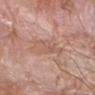Impression:
Imaged during a routine full-body skin examination; the lesion was not biopsied and no histopathology is available.
Background:
Imaged with white-light lighting. A lesion tile, about 15 mm wide, cut from a 3D total-body photograph. Located on the right forearm. Automated tile analysis of the lesion measured an area of roughly 7.5 mm², an eccentricity of roughly 0.9, and a symmetry-axis asymmetry near 0.3. It also reported a mean CIELAB color near L≈58 a*≈21 b*≈27, about 7 CIELAB-L* units darker than the surrounding skin, and a lesion-to-skin contrast of about 5 (normalized; higher = more distinct). The analysis additionally found a border-irregularity rating of about 5/10, a within-lesion color-variation index near 3/10, and peripheral color asymmetry of about 1. And it measured a classifier nevus-likeness of about 0/100. A male subject, approximately 60 years of age. The recorded lesion diameter is about 5 mm.